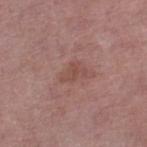notes=catalogued during a skin exam; not biopsied
site=the right lower leg
acquisition=total-body-photography crop, ~15 mm field of view
subject=female, aged around 60